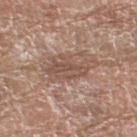Notes:
* notes · no biopsy performed (imaged during a skin exam)
* size · ≈5.5 mm
* image · ~15 mm crop, total-body skin-cancer survey
* subject · female, in their mid- to late 70s
* location · the left thigh